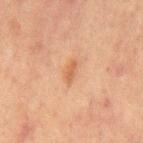biopsy status=no biopsy performed (imaged during a skin exam)
tile lighting=cross-polarized illumination
anatomic site=the chest
imaging modality=~15 mm tile from a whole-body skin photo
image-analysis metrics=an area of roughly 3 mm², a shape eccentricity near 0.9, and two-axis asymmetry of about 0.3; a classifier nevus-likeness of about 25/100
lesion diameter=about 3 mm
subject=male, approximately 65 years of age